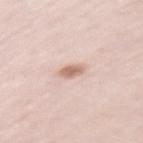Recorded during total-body skin imaging; not selected for excision or biopsy.
A female patient roughly 65 years of age.
A close-up tile cropped from a whole-body skin photograph, about 15 mm across.
Captured under white-light illumination.
The lesion is on the left upper arm.
Approximately 2.5 mm at its widest.